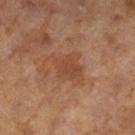This lesion was catalogued during total-body skin photography and was not selected for biopsy. Measured at roughly 5 mm in maximum diameter. The lesion is located on the right lower leg. Automated image analysis of the tile measured an eccentricity of roughly 0.75 and two-axis asymmetry of about 0.35. The analysis additionally found roughly 6 lightness units darker than nearby skin and a lesion-to-skin contrast of about 5.5 (normalized; higher = more distinct). It also reported radial color variation of about 0.5. This image is a 15 mm lesion crop taken from a total-body photograph. A female subject, roughly 60 years of age.